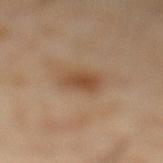Recorded during total-body skin imaging; not selected for excision or biopsy. Located on the left lower leg. A male patient aged around 55. A 15 mm close-up extracted from a 3D total-body photography capture. About 3 mm across.